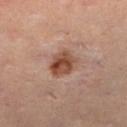Captured during whole-body skin photography for melanoma surveillance; the lesion was not biopsied.
A female patient aged around 35.
This image is a 15 mm lesion crop taken from a total-body photograph.
Automated image analysis of the tile measured a shape eccentricity near 0.3 and a shape-asymmetry score of about 0.15 (0 = symmetric). And it measured a mean CIELAB color near L≈46 a*≈22 b*≈29, about 12 CIELAB-L* units darker than the surrounding skin, and a lesion-to-skin contrast of about 9.5 (normalized; higher = more distinct). And it measured a border-irregularity index near 1.5/10. And it measured a nevus-likeness score of about 95/100 and a detector confidence of about 100 out of 100 that the crop contains a lesion.
On the left lower leg.
This is a cross-polarized tile.